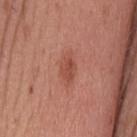notes: total-body-photography surveillance lesion; no biopsy | body site: the head or neck | subject: male, approximately 25 years of age | imaging modality: ~15 mm tile from a whole-body skin photo.